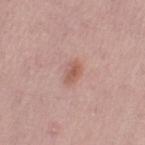Clinical impression: The lesion was tiled from a total-body skin photograph and was not biopsied. Clinical summary: An algorithmic analysis of the crop reported a footprint of about 4 mm² and a symmetry-axis asymmetry near 0.2. And it measured a border-irregularity index near 2/10 and a peripheral color-asymmetry measure near 1. From the left thigh. The recorded lesion diameter is about 3 mm. A female patient, aged around 50. A 15 mm close-up tile from a total-body photography series done for melanoma screening.